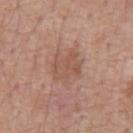Notes:
• follow-up · no biopsy performed (imaged during a skin exam)